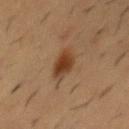  biopsy_status: not biopsied; imaged during a skin examination
  lesion_size:
    long_diameter_mm_approx: 4.5
  automated_metrics:
    cielab_L: 34
    cielab_a: 17
    cielab_b: 29
    vs_skin_darker_L: 10.0
    nevus_likeness_0_100: 100
  image:
    source: total-body photography crop
    field_of_view_mm: 15
  patient:
    sex: male
    age_approx: 55
  lighting: cross-polarized
  site: mid back